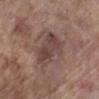{
  "biopsy_status": "not biopsied; imaged during a skin examination",
  "site": "left lower leg",
  "lesion_size": {
    "long_diameter_mm_approx": 6.0
  },
  "image": {
    "source": "total-body photography crop",
    "field_of_view_mm": 15
  },
  "lighting": "white-light",
  "patient": {
    "sex": "female",
    "age_approx": "85+"
  },
  "automated_metrics": {
    "nevus_likeness_0_100": 0,
    "lesion_detection_confidence_0_100": 100
  }
}